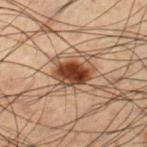Q: Is there a histopathology result?
A: catalogued during a skin exam; not biopsied
Q: What did automated image analysis measure?
A: a nevus-likeness score of about 100/100
Q: What is the anatomic site?
A: the left lower leg
Q: How large is the lesion?
A: ≈4 mm
Q: How was this image acquired?
A: ~15 mm tile from a whole-body skin photo
Q: Who is the patient?
A: male, approximately 50 years of age
Q: Illumination type?
A: cross-polarized illumination